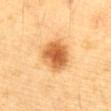Impression:
The lesion was tiled from a total-body skin photograph and was not biopsied.
Background:
A 15 mm close-up extracted from a 3D total-body photography capture. The patient is a male in their mid-80s or older. This is a cross-polarized tile. The lesion is located on the abdomen.